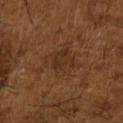<lesion>
  <biopsy_status>not biopsied; imaged during a skin examination</biopsy_status>
  <lesion_size>
    <long_diameter_mm_approx>5.5</long_diameter_mm_approx>
  </lesion_size>
  <patient>
    <sex>male</sex>
    <age_approx>65</age_approx>
  </patient>
  <image>
    <source>total-body photography crop</source>
    <field_of_view_mm>15</field_of_view_mm>
  </image>
  <site>right upper arm</site>
  <lighting>cross-polarized</lighting>
  <automated_metrics>
    <area_mm2_approx>14.0</area_mm2_approx>
    <eccentricity>0.55</eccentricity>
    <nevus_likeness_0_100>0</nevus_likeness_0_100>
  </automated_metrics>
</lesion>